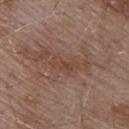Clinical impression:
The lesion was tiled from a total-body skin photograph and was not biopsied.
Background:
A region of skin cropped from a whole-body photographic capture, roughly 15 mm wide. From the upper back. Imaged with white-light lighting. The recorded lesion diameter is about 3.5 mm. A male patient approximately 55 years of age.